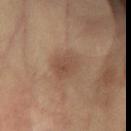| feature | finding |
|---|---|
| patient | female, about 60 years old |
| lesion diameter | ~3.5 mm (longest diameter) |
| automated lesion analysis | an eccentricity of roughly 0.75 and a shape-asymmetry score of about 0.15 (0 = symmetric); an average lesion color of about L≈49 a*≈18 b*≈29 (CIELAB), roughly 8 lightness units darker than nearby skin, and a normalized lesion–skin contrast near 6; a border-irregularity rating of about 1.5/10, internal color variation of about 3 on a 0–10 scale, and radial color variation of about 1; a nevus-likeness score of about 5/100 and a lesion-detection confidence of about 100/100 |
| anatomic site | the left arm |
| image source | 15 mm crop, total-body photography |